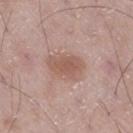Assessment:
No biopsy was performed on this lesion — it was imaged during a full skin examination and was not determined to be concerning.
Image and clinical context:
The patient is a male aged approximately 50. A 15 mm close-up extracted from a 3D total-body photography capture. Located on the right thigh. Measured at roughly 4.5 mm in maximum diameter. Automated image analysis of the tile measured a shape-asymmetry score of about 0.15 (0 = symmetric). And it measured an average lesion color of about L≈56 a*≈19 b*≈25 (CIELAB), about 9 CIELAB-L* units darker than the surrounding skin, and a lesion-to-skin contrast of about 6.5 (normalized; higher = more distinct). And it measured a border-irregularity rating of about 2/10 and a peripheral color-asymmetry measure near 0.5.